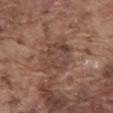Part of a total-body skin-imaging series; this lesion was reviewed on a skin check and was not flagged for biopsy. The total-body-photography lesion software estimated a mean CIELAB color near L≈44 a*≈18 b*≈25, about 7 CIELAB-L* units darker than the surrounding skin, and a normalized lesion–skin contrast near 5.5. It also reported border irregularity of about 3.5 on a 0–10 scale. The analysis additionally found a nevus-likeness score of about 0/100 and lesion-presence confidence of about 75/100. The tile uses white-light illumination. A lesion tile, about 15 mm wide, cut from a 3D total-body photograph. A male subject in their mid-70s. From the abdomen.